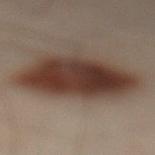Clinical impression: The lesion was photographed on a routine skin check and not biopsied; there is no pathology result. Acquisition and patient details: A male patient about 50 years old. Captured under cross-polarized illumination. Located on the left lower leg. A close-up tile cropped from a whole-body skin photograph, about 15 mm across. About 8 mm across.